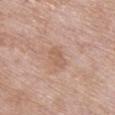biopsy status: no biopsy performed (imaged during a skin exam)
image-analysis metrics: an area of roughly 4 mm², a shape eccentricity near 0.85, and a symmetry-axis asymmetry near 0.25; a lesion–skin lightness drop of about 7 and a normalized border contrast of about 5
size: ~3 mm (longest diameter)
lighting: white-light illumination
patient: female, in their 70s
imaging modality: ~15 mm tile from a whole-body skin photo
anatomic site: the chest